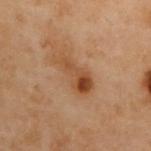This is a cross-polarized tile.
Measured at roughly 5 mm in maximum diameter.
Located on the back.
A female patient, aged 58 to 62.
Cropped from a whole-body photographic skin survey; the tile spans about 15 mm.
The total-body-photography lesion software estimated a lesion color around L≈42 a*≈19 b*≈32 in CIELAB, roughly 8 lightness units darker than nearby skin, and a lesion-to-skin contrast of about 7.5 (normalized; higher = more distinct).